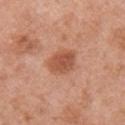Findings:
• notes: total-body-photography surveillance lesion; no biopsy
• acquisition: total-body-photography crop, ~15 mm field of view
• anatomic site: the right upper arm
• subject: female, aged 48–52